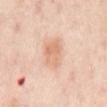notes — imaged on a skin check; not biopsied
image source — ~15 mm tile from a whole-body skin photo
site — the abdomen
subject — roughly 55 years of age